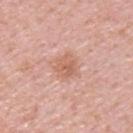site — the upper back; patient — male, aged 48–52; lesion diameter — ≈3 mm; image — ~15 mm crop, total-body skin-cancer survey; illumination — white-light illumination; image-analysis metrics — a border-irregularity rating of about 2.5/10, internal color variation of about 3 on a 0–10 scale, and radial color variation of about 1.